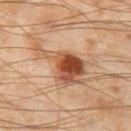This lesion was catalogued during total-body skin photography and was not selected for biopsy.
A male patient roughly 45 years of age.
A roughly 15 mm field-of-view crop from a total-body skin photograph.
An algorithmic analysis of the crop reported an area of roughly 17 mm², a shape eccentricity near 0.85, and a symmetry-axis asymmetry near 0.5.
On the left thigh.
Measured at roughly 7.5 mm in maximum diameter.
This is a cross-polarized tile.